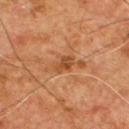Acquisition and patient details: A 15 mm crop from a total-body photograph taken for skin-cancer surveillance. A male subject in their mid- to late 50s. Approximately 2.5 mm at its widest. From the chest.